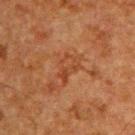<tbp_lesion>
  <biopsy_status>not biopsied; imaged during a skin examination</biopsy_status>
  <lesion_size>
    <long_diameter_mm_approx>3.0</long_diameter_mm_approx>
  </lesion_size>
  <lighting>cross-polarized</lighting>
  <automated_metrics>
    <eccentricity>0.75</eccentricity>
    <shape_asymmetry>0.4</shape_asymmetry>
    <border_irregularity_0_10>5.0</border_irregularity_0_10>
    <color_variation_0_10>3.0</color_variation_0_10>
    <peripheral_color_asymmetry>1.0</peripheral_color_asymmetry>
    <nevus_likeness_0_100>0</nevus_likeness_0_100>
    <lesion_detection_confidence_0_100>95</lesion_detection_confidence_0_100>
  </automated_metrics>
  <site>upper back</site>
  <patient>
    <sex>male</sex>
    <age_approx>60</age_approx>
  </patient>
  <image>
    <source>total-body photography crop</source>
    <field_of_view_mm>15</field_of_view_mm>
  </image>
</tbp_lesion>